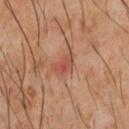follow-up=total-body-photography surveillance lesion; no biopsy
subject=male, aged 58 to 62
location=the front of the torso
imaging modality=total-body-photography crop, ~15 mm field of view